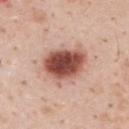follow-up: catalogued during a skin exam; not biopsied